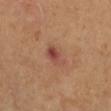Q: Is there a histopathology result?
A: imaged on a skin check; not biopsied
Q: What kind of image is this?
A: ~15 mm tile from a whole-body skin photo
Q: What is the lesion's diameter?
A: ~3 mm (longest diameter)
Q: Patient demographics?
A: female, approximately 65 years of age
Q: Illumination type?
A: cross-polarized
Q: Lesion location?
A: the right upper arm
Q: Automated lesion metrics?
A: a shape eccentricity near 0.8 and two-axis asymmetry of about 0.25; a lesion color around L≈46 a*≈25 b*≈27 in CIELAB and a normalized border contrast of about 7.5; internal color variation of about 8 on a 0–10 scale and peripheral color asymmetry of about 3; a classifier nevus-likeness of about 0/100 and a lesion-detection confidence of about 100/100